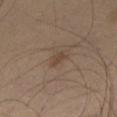Q: Lesion size?
A: about 2.5 mm
Q: How was this image acquired?
A: total-body-photography crop, ~15 mm field of view
Q: Where on the body is the lesion?
A: the right thigh
Q: Illumination type?
A: cross-polarized